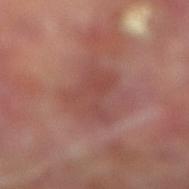Impression: No biopsy was performed on this lesion — it was imaged during a full skin examination and was not determined to be concerning. Image and clinical context: A close-up tile cropped from a whole-body skin photograph, about 15 mm across. This is a cross-polarized tile. Approximately 5.5 mm at its widest. A male subject, aged approximately 70. The lesion is located on the right lower leg.